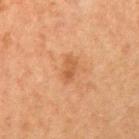Clinical impression:
Captured during whole-body skin photography for melanoma surveillance; the lesion was not biopsied.
Context:
This is a cross-polarized tile. The lesion's longest dimension is about 2.5 mm. A close-up tile cropped from a whole-body skin photograph, about 15 mm across. The subject is a female roughly 40 years of age. The lesion is on the right upper arm.